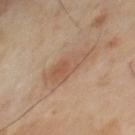workup — no biopsy performed (imaged during a skin exam) | subject — male, about 70 years old | image source — ~15 mm crop, total-body skin-cancer survey | tile lighting — cross-polarized illumination | size — ≈5.5 mm | site — the chest.